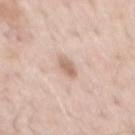notes = catalogued during a skin exam; not biopsied
image source = 15 mm crop, total-body photography
location = the back
size = about 3 mm
subject = male, roughly 60 years of age
tile lighting = white-light illumination